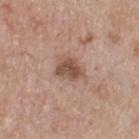Clinical impression:
The lesion was photographed on a routine skin check and not biopsied; there is no pathology result.
Image and clinical context:
A close-up tile cropped from a whole-body skin photograph, about 15 mm across. The recorded lesion diameter is about 3.5 mm. The subject is a male in their mid-50s.